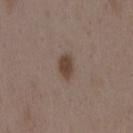  biopsy_status: not biopsied; imaged during a skin examination
  site: mid back
  lesion_size:
    long_diameter_mm_approx: 3.0
  image:
    source: total-body photography crop
    field_of_view_mm: 15
  automated_metrics:
    cielab_L: 42
    cielab_a: 14
    cielab_b: 24
    vs_skin_contrast_norm: 9.0
    border_irregularity_0_10: 1.0
    color_variation_0_10: 1.5
    peripheral_color_asymmetry: 0.5
  patient:
    sex: female
    age_approx: 35
  lighting: white-light